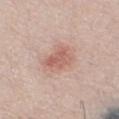This lesion was catalogued during total-body skin photography and was not selected for biopsy. The total-body-photography lesion software estimated an eccentricity of roughly 0.65. The software also gave an average lesion color of about L≈60 a*≈24 b*≈26 (CIELAB) and a lesion-to-skin contrast of about 6.5 (normalized; higher = more distinct). The software also gave a border-irregularity index near 3.5/10, a within-lesion color-variation index near 4.5/10, and a peripheral color-asymmetry measure near 1.5. This image is a 15 mm lesion crop taken from a total-body photograph. A male subject, aged 28 to 32. Imaged with white-light lighting. The recorded lesion diameter is about 3.5 mm.